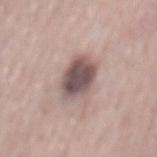Recorded during total-body skin imaging; not selected for excision or biopsy.
Longest diameter approximately 5 mm.
The patient is a male about 65 years old.
A 15 mm close-up extracted from a 3D total-body photography capture.
From the mid back.